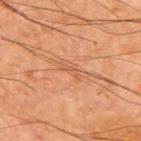<case>
  <biopsy_status>not biopsied; imaged during a skin examination</biopsy_status>
  <lighting>cross-polarized</lighting>
  <site>upper back</site>
  <lesion_size>
    <long_diameter_mm_approx>2.5</long_diameter_mm_approx>
  </lesion_size>
  <image>
    <source>total-body photography crop</source>
    <field_of_view_mm>15</field_of_view_mm>
  </image>
  <patient>
    <sex>male</sex>
    <age_approx>65</age_approx>
  </patient>
</case>